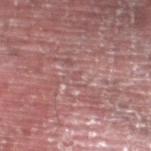Case summary:
* notes: catalogued during a skin exam; not biopsied
* lesion diameter: ≈1 mm
* subject: male, about 55 years old
* image-analysis metrics: an average lesion color of about L≈45 a*≈20 b*≈17 (CIELAB) and roughly 4 lightness units darker than nearby skin; a within-lesion color-variation index near 0/10; lesion-presence confidence of about 0/100
* imaging modality: ~15 mm tile from a whole-body skin photo
* location: the left lower leg